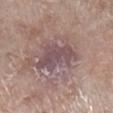This lesion was catalogued during total-body skin photography and was not selected for biopsy. The patient is a female roughly 75 years of age. From the right lower leg. About 6 mm across. The lesion-visualizer software estimated a lesion area of about 14 mm², an outline eccentricity of about 0.8 (0 = round, 1 = elongated), and a symmetry-axis asymmetry near 0.4. It also reported border irregularity of about 5 on a 0–10 scale, a color-variation rating of about 3.5/10, and radial color variation of about 1. And it measured an automated nevus-likeness rating near 0 out of 100. A roughly 15 mm field-of-view crop from a total-body skin photograph.